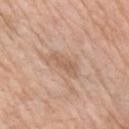Q: Was a biopsy performed?
A: imaged on a skin check; not biopsied
Q: Automated lesion metrics?
A: a border-irregularity index near 4.5/10, internal color variation of about 1.5 on a 0–10 scale, and radial color variation of about 0.5
Q: What is the imaging modality?
A: ~15 mm tile from a whole-body skin photo
Q: Who is the patient?
A: female, roughly 65 years of age
Q: Lesion size?
A: ~4 mm (longest diameter)
Q: Where on the body is the lesion?
A: the arm